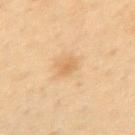Impression:
The lesion was photographed on a routine skin check and not biopsied; there is no pathology result.
Context:
The lesion is located on the upper back. A close-up tile cropped from a whole-body skin photograph, about 15 mm across. The tile uses cross-polarized illumination. Longest diameter approximately 2 mm. A male patient approximately 55 years of age.